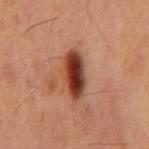biopsy_status: not biopsied; imaged during a skin examination
lighting: cross-polarized
lesion_size:
  long_diameter_mm_approx: 5.0
image:
  source: total-body photography crop
  field_of_view_mm: 15
patient:
  sex: male
  age_approx: 60
site: back